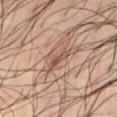This lesion was catalogued during total-body skin photography and was not selected for biopsy. The subject is a male aged approximately 35. On the abdomen. Measured at roughly 3 mm in maximum diameter. Captured under cross-polarized illumination. A roughly 15 mm field-of-view crop from a total-body skin photograph.